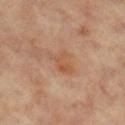Findings:
* biopsy status — imaged on a skin check; not biopsied
* acquisition — ~15 mm tile from a whole-body skin photo
* illumination — cross-polarized illumination
* subject — female, about 65 years old
* location — the left leg
* size — ~3.5 mm (longest diameter)